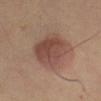Q: Was this lesion biopsied?
A: imaged on a skin check; not biopsied
Q: What did automated image analysis measure?
A: an area of roughly 20 mm², a shape eccentricity near 0.6, and a shape-asymmetry score of about 0.15 (0 = symmetric); a nevus-likeness score of about 95/100 and a lesion-detection confidence of about 100/100
Q: What lighting was used for the tile?
A: cross-polarized
Q: What are the patient's age and sex?
A: female, roughly 60 years of age
Q: How large is the lesion?
A: about 5.5 mm
Q: What kind of image is this?
A: ~15 mm tile from a whole-body skin photo
Q: Where on the body is the lesion?
A: the right leg